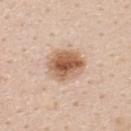This lesion was catalogued during total-body skin photography and was not selected for biopsy. A 15 mm crop from a total-body photograph taken for skin-cancer surveillance. Located on the upper back. A female patient approximately 45 years of age. This is a white-light tile.